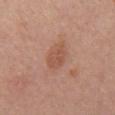Q: Was this lesion biopsied?
A: total-body-photography surveillance lesion; no biopsy
Q: Illumination type?
A: white-light illumination
Q: Where on the body is the lesion?
A: the chest
Q: Patient demographics?
A: male, aged around 65
Q: What did automated image analysis measure?
A: a lesion area of about 5.5 mm² and an outline eccentricity of about 0.7 (0 = round, 1 = elongated); a lesion color around L≈53 a*≈23 b*≈30 in CIELAB and a lesion-to-skin contrast of about 6 (normalized; higher = more distinct); a within-lesion color-variation index near 2/10 and peripheral color asymmetry of about 0.5
Q: Lesion size?
A: about 3.5 mm
Q: What kind of image is this?
A: 15 mm crop, total-body photography